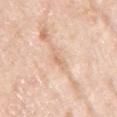Recorded during total-body skin imaging; not selected for excision or biopsy. Longest diameter approximately 4 mm. This is a white-light tile. A roughly 15 mm field-of-view crop from a total-body skin photograph. Automated tile analysis of the lesion measured a shape eccentricity near 0.9 and two-axis asymmetry of about 0.25. The software also gave a lesion color around L≈72 a*≈18 b*≈32 in CIELAB and a normalized border contrast of about 5. A female subject approximately 70 years of age. The lesion is located on the left upper arm.